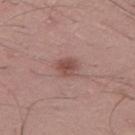Part of a total-body skin-imaging series; this lesion was reviewed on a skin check and was not flagged for biopsy. The lesion is located on the left thigh. An algorithmic analysis of the crop reported a lesion color around L≈49 a*≈22 b*≈23 in CIELAB and roughly 10 lightness units darker than nearby skin. The software also gave a nevus-likeness score of about 70/100 and a detector confidence of about 100 out of 100 that the crop contains a lesion. A 15 mm close-up tile from a total-body photography series done for melanoma screening. Measured at roughly 2.5 mm in maximum diameter. Imaged with white-light lighting. The subject is a male aged 18–22.